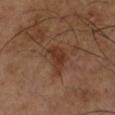- follow-up: imaged on a skin check; not biopsied
- location: the leg
- patient: male, aged approximately 65
- lighting: cross-polarized illumination
- lesion diameter: about 3.5 mm
- image: ~15 mm crop, total-body skin-cancer survey